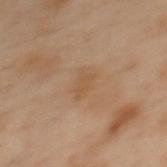This lesion was catalogued during total-body skin photography and was not selected for biopsy.
A region of skin cropped from a whole-body photographic capture, roughly 15 mm wide.
About 3 mm across.
Imaged with cross-polarized lighting.
A female patient, aged 53 to 57.
The lesion is located on the upper back.